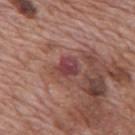Q: Is there a histopathology result?
A: imaged on a skin check; not biopsied
Q: How was this image acquired?
A: total-body-photography crop, ~15 mm field of view
Q: Lesion location?
A: the mid back
Q: What is the lesion's diameter?
A: ~3 mm (longest diameter)
Q: How was the tile lit?
A: white-light
Q: Who is the patient?
A: male, aged 68–72